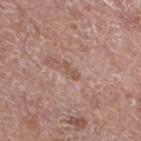biopsy status: total-body-photography surveillance lesion; no biopsy
diameter: ≈2.5 mm
automated lesion analysis: border irregularity of about 5 on a 0–10 scale, a within-lesion color-variation index near 0/10, and peripheral color asymmetry of about 0; a classifier nevus-likeness of about 0/100 and a detector confidence of about 95 out of 100 that the crop contains a lesion
acquisition: ~15 mm crop, total-body skin-cancer survey
anatomic site: the right lower leg
tile lighting: white-light
subject: male, about 60 years old